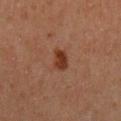follow-up = no biopsy performed (imaged during a skin exam) | patient = male, about 65 years old | lesion size = ≈3 mm | tile lighting = cross-polarized | image source = ~15 mm crop, total-body skin-cancer survey | site = the back.